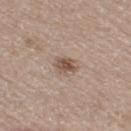Assessment: Imaged during a routine full-body skin examination; the lesion was not biopsied and no histopathology is available. Context: Automated tile analysis of the lesion measured an area of roughly 5.5 mm², an outline eccentricity of about 0.5 (0 = round, 1 = elongated), and a symmetry-axis asymmetry near 0.15. The software also gave a mean CIELAB color near L≈53 a*≈15 b*≈26 and a normalized border contrast of about 7.5. A female patient, in their 70s. A 15 mm close-up tile from a total-body photography series done for melanoma screening. The lesion is located on the leg. The lesion's longest dimension is about 3 mm.